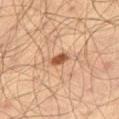Q: Is there a histopathology result?
A: catalogued during a skin exam; not biopsied
Q: What did automated image analysis measure?
A: a lesion area of about 3 mm² and a symmetry-axis asymmetry near 0.15; a lesion color around L≈44 a*≈21 b*≈30 in CIELAB and about 12 CIELAB-L* units darker than the surrounding skin; a border-irregularity rating of about 1.5/10, internal color variation of about 1.5 on a 0–10 scale, and peripheral color asymmetry of about 0.5; a detector confidence of about 100 out of 100 that the crop contains a lesion
Q: What is the lesion's diameter?
A: ~2.5 mm (longest diameter)
Q: What is the imaging modality?
A: ~15 mm crop, total-body skin-cancer survey
Q: Lesion location?
A: the leg
Q: Patient demographics?
A: male, aged 43 to 47